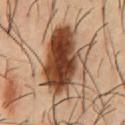| field | value |
|---|---|
| workup | imaged on a skin check; not biopsied |
| acquisition | ~15 mm crop, total-body skin-cancer survey |
| lighting | cross-polarized illumination |
| body site | the chest |
| lesion diameter | about 9 mm |
| automated metrics | a lesion area of about 33 mm² and an eccentricity of roughly 0.8; an average lesion color of about L≈43 a*≈20 b*≈31 (CIELAB) and a lesion–skin lightness drop of about 20; a nevus-likeness score of about 100/100 and a detector confidence of about 100 out of 100 that the crop contains a lesion |
| patient | male, roughly 55 years of age |